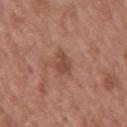No biopsy was performed on this lesion — it was imaged during a full skin examination and was not determined to be concerning. Captured under white-light illumination. Measured at roughly 2.5 mm in maximum diameter. A male subject aged 48–52. Automated tile analysis of the lesion measured a nevus-likeness score of about 0/100 and a lesion-detection confidence of about 100/100. From the mid back. Cropped from a whole-body photographic skin survey; the tile spans about 15 mm.